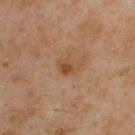Assessment:
Recorded during total-body skin imaging; not selected for excision or biopsy.
Acquisition and patient details:
This is a cross-polarized tile. From the back. A male patient aged 53–57. Approximately 2.5 mm at its widest. A 15 mm crop from a total-body photograph taken for skin-cancer surveillance.